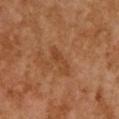illumination: cross-polarized; image: ~15 mm tile from a whole-body skin photo; size: ~3.5 mm (longest diameter); anatomic site: the chest; subject: female, aged 58 to 62; image-analysis metrics: a lesion area of about 3.5 mm², a shape eccentricity near 0.95, and a symmetry-axis asymmetry near 0.55.